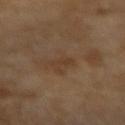Part of a total-body skin-imaging series; this lesion was reviewed on a skin check and was not flagged for biopsy. A female patient, roughly 70 years of age. Captured under cross-polarized illumination. Located on the right forearm. The recorded lesion diameter is about 3 mm. A roughly 15 mm field-of-view crop from a total-body skin photograph.